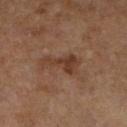follow-up: total-body-photography surveillance lesion; no biopsy
site: the right lower leg
patient: female, approximately 60 years of age
image: total-body-photography crop, ~15 mm field of view
size: about 4 mm
lighting: cross-polarized illumination
image-analysis metrics: an automated nevus-likeness rating near 0 out of 100 and a detector confidence of about 100 out of 100 that the crop contains a lesion